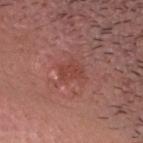Case summary:
• follow-up · no biopsy performed (imaged during a skin exam)
• subject · male, aged approximately 40
• image · total-body-photography crop, ~15 mm field of view
• location · the head or neck